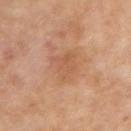The lesion was photographed on a routine skin check and not biopsied; there is no pathology result.
Captured under cross-polarized illumination.
A close-up tile cropped from a whole-body skin photograph, about 15 mm across.
About 4 mm across.
A male subject, in their mid-60s.
Automated tile analysis of the lesion measured a lesion color around L≈54 a*≈21 b*≈33 in CIELAB. The analysis additionally found a nevus-likeness score of about 0/100 and a detector confidence of about 100 out of 100 that the crop contains a lesion.